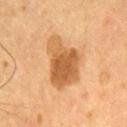Imaged during a routine full-body skin examination; the lesion was not biopsied and no histopathology is available.
The lesion is on the chest.
This image is a 15 mm lesion crop taken from a total-body photograph.
A male subject aged 53 to 57.
This is a cross-polarized tile.
The lesion's longest dimension is about 6.5 mm.